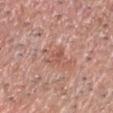Assessment:
Part of a total-body skin-imaging series; this lesion was reviewed on a skin check and was not flagged for biopsy.
Acquisition and patient details:
The subject is a male aged 58–62. The lesion's longest dimension is about 3.5 mm. The lesion is on the head or neck. Imaged with white-light lighting. A close-up tile cropped from a whole-body skin photograph, about 15 mm across.